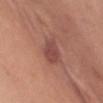| field | value |
|---|---|
| image source | total-body-photography crop, ~15 mm field of view |
| illumination | white-light |
| subject | female, about 45 years old |
| site | the chest |
| size | about 3.5 mm |